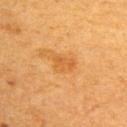image:
  source: total-body photography crop
  field_of_view_mm: 15
lighting: cross-polarized
patient:
  sex: female
  age_approx: 55
site: upper back